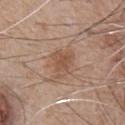Context:
Imaged with white-light lighting. A 15 mm crop from a total-body photograph taken for skin-cancer surveillance. Automated tile analysis of the lesion measured a color-variation rating of about 3/10 and peripheral color asymmetry of about 1. The analysis additionally found lesion-presence confidence of about 100/100. The lesion's longest dimension is about 3.5 mm. The lesion is on the front of the torso. A male subject roughly 65 years of age.